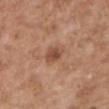Case summary:
- notes — imaged on a skin check; not biopsied
- patient — male, in their mid-50s
- lesion diameter — ≈2.5 mm
- illumination — white-light
- anatomic site — the left upper arm
- image — ~15 mm crop, total-body skin-cancer survey
- automated lesion analysis — an outline eccentricity of about 0.5 (0 = round, 1 = elongated) and a symmetry-axis asymmetry near 0.2; a lesion–skin lightness drop of about 10 and a lesion-to-skin contrast of about 7.5 (normalized; higher = more distinct)